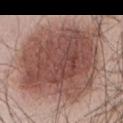The patient is a male in their mid-50s. A lesion tile, about 15 mm wide, cut from a 3D total-body photograph. Measured at roughly 11.5 mm in maximum diameter. From the abdomen. An algorithmic analysis of the crop reported a lesion area of about 80 mm², a shape eccentricity near 0.55, and a symmetry-axis asymmetry near 0.15. The analysis additionally found a lesion color around L≈49 a*≈21 b*≈24 in CIELAB and a lesion–skin lightness drop of about 14. Captured under white-light illumination.